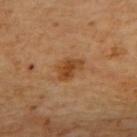Q: Was a biopsy performed?
A: no biopsy performed (imaged during a skin exam)
Q: Automated lesion metrics?
A: an area of roughly 6.5 mm², an outline eccentricity of about 0.7 (0 = round, 1 = elongated), and two-axis asymmetry of about 0.3; an average lesion color of about L≈44 a*≈22 b*≈37 (CIELAB), a lesion–skin lightness drop of about 9, and a normalized border contrast of about 8; an automated nevus-likeness rating near 80 out of 100 and a detector confidence of about 100 out of 100 that the crop contains a lesion
Q: Patient demographics?
A: aged 68 to 72
Q: What lighting was used for the tile?
A: cross-polarized
Q: Where on the body is the lesion?
A: the upper back
Q: What is the imaging modality?
A: ~15 mm crop, total-body skin-cancer survey
Q: Lesion size?
A: ≈3.5 mm